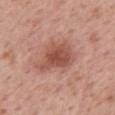Captured during whole-body skin photography for melanoma surveillance; the lesion was not biopsied. Imaged with white-light lighting. Longest diameter approximately 5.5 mm. A lesion tile, about 15 mm wide, cut from a 3D total-body photograph. The lesion-visualizer software estimated a footprint of about 14 mm², an eccentricity of roughly 0.75, and a symmetry-axis asymmetry near 0.35. The software also gave border irregularity of about 4 on a 0–10 scale, internal color variation of about 3.5 on a 0–10 scale, and a peripheral color-asymmetry measure near 1. A female subject aged around 65. On the upper back.